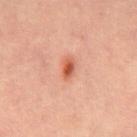Q: Was this lesion biopsied?
A: catalogued during a skin exam; not biopsied
Q: What is the imaging modality?
A: ~15 mm crop, total-body skin-cancer survey
Q: Patient demographics?
A: male, approximately 45 years of age
Q: What is the anatomic site?
A: the abdomen
Q: Automated lesion metrics?
A: an outline eccentricity of about 0.8 (0 = round, 1 = elongated) and a shape-asymmetry score of about 0.3 (0 = symmetric); a detector confidence of about 100 out of 100 that the crop contains a lesion
Q: How was the tile lit?
A: cross-polarized illumination